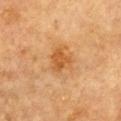Imaged during a routine full-body skin examination; the lesion was not biopsied and no histopathology is available.
This image is a 15 mm lesion crop taken from a total-body photograph.
Measured at roughly 4 mm in maximum diameter.
This is a cross-polarized tile.
On the front of the torso.
A male patient in their mid- to late 80s or older.